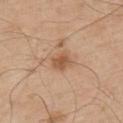Recorded during total-body skin imaging; not selected for excision or biopsy.
Imaged with white-light lighting.
A male subject, aged approximately 55.
The lesion is on the upper back.
A lesion tile, about 15 mm wide, cut from a 3D total-body photograph.